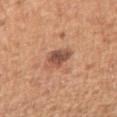– workup — catalogued during a skin exam; not biopsied
– tile lighting — white-light
– automated lesion analysis — an outline eccentricity of about 0.6 (0 = round, 1 = elongated) and a shape-asymmetry score of about 0.3 (0 = symmetric); a lesion color around L≈52 a*≈23 b*≈30 in CIELAB, a lesion–skin lightness drop of about 12, and a normalized border contrast of about 8.5; a nevus-likeness score of about 45/100
– acquisition — total-body-photography crop, ~15 mm field of view
– anatomic site — the right upper arm
– patient — male, in their mid-50s